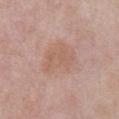<case>
<biopsy_status>not biopsied; imaged during a skin examination</biopsy_status>
<site>abdomen</site>
<image>
  <source>total-body photography crop</source>
  <field_of_view_mm>15</field_of_view_mm>
</image>
<patient>
  <sex>male</sex>
  <age_approx>55</age_approx>
</patient>
</case>